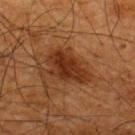The lesion was tiled from a total-body skin photograph and was not biopsied.
The lesion is on the upper back.
A male subject about 65 years old.
The lesion's longest dimension is about 5.5 mm.
Cropped from a whole-body photographic skin survey; the tile spans about 15 mm.